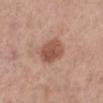Imaged during a routine full-body skin examination; the lesion was not biopsied and no histopathology is available.
A female subject in their mid-60s.
About 3.5 mm across.
A roughly 15 mm field-of-view crop from a total-body skin photograph.
Captured under white-light illumination.
The lesion is located on the left lower leg.